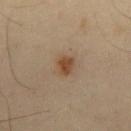Q: Is there a histopathology result?
A: catalogued during a skin exam; not biopsied